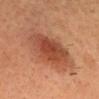Case summary:
- follow-up — total-body-photography surveillance lesion; no biopsy
- patient — male, aged 38–42
- lighting — cross-polarized illumination
- lesion diameter — ≈5.5 mm
- anatomic site — the head or neck
- image source — ~15 mm tile from a whole-body skin photo